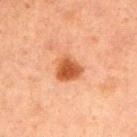| field | value |
|---|---|
| workup | imaged on a skin check; not biopsied |
| acquisition | ~15 mm tile from a whole-body skin photo |
| site | the left upper arm |
| subject | male, about 60 years old |